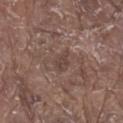Assessment: Recorded during total-body skin imaging; not selected for excision or biopsy. Background: A male patient, about 80 years old. A region of skin cropped from a whole-body photographic capture, roughly 15 mm wide. The tile uses white-light illumination. An algorithmic analysis of the crop reported an eccentricity of roughly 0.75 and a shape-asymmetry score of about 0.3 (0 = symmetric). The software also gave a classifier nevus-likeness of about 0/100 and lesion-presence confidence of about 75/100. The lesion is located on the right lower leg. Measured at roughly 3 mm in maximum diameter.